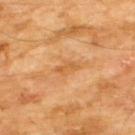Findings:
– notes · no biopsy performed (imaged during a skin exam)
– anatomic site · the chest
– lighting · cross-polarized illumination
– patient · male, approximately 60 years of age
– lesion size · about 3 mm
– image source · total-body-photography crop, ~15 mm field of view